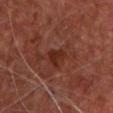Q: Was this lesion biopsied?
A: no biopsy performed (imaged during a skin exam)